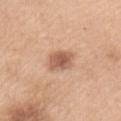Impression:
This lesion was catalogued during total-body skin photography and was not selected for biopsy.
Image and clinical context:
The patient is a female in their mid-50s. The lesion is located on the left upper arm. Captured under white-light illumination. A region of skin cropped from a whole-body photographic capture, roughly 15 mm wide.